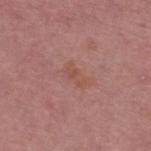The lesion was tiled from a total-body skin photograph and was not biopsied. The recorded lesion diameter is about 3 mm. On the upper back. A close-up tile cropped from a whole-body skin photograph, about 15 mm across. A male subject, approximately 55 years of age.